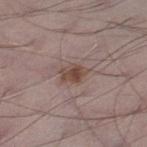Context: A male patient, about 70 years old. The tile uses white-light illumination. An algorithmic analysis of the crop reported a mean CIELAB color near L≈46 a*≈17 b*≈23, roughly 10 lightness units darker than nearby skin, and a lesion-to-skin contrast of about 8.5 (normalized; higher = more distinct). The software also gave border irregularity of about 3 on a 0–10 scale, a within-lesion color-variation index near 4.5/10, and peripheral color asymmetry of about 1.5. It also reported a nevus-likeness score of about 80/100 and a lesion-detection confidence of about 100/100. The lesion is located on the leg. A 15 mm crop from a total-body photograph taken for skin-cancer surveillance. About 3 mm across.